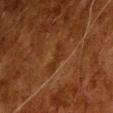{"biopsy_status": "not biopsied; imaged during a skin examination", "site": "upper back", "image": {"source": "total-body photography crop", "field_of_view_mm": 15}, "lesion_size": {"long_diameter_mm_approx": 3.5}, "patient": {"sex": "male", "age_approx": 80}}